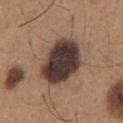notes=no biopsy performed (imaged during a skin exam); body site=the chest; TBP lesion metrics=a border-irregularity rating of about 1.5/10, a color-variation rating of about 6/10, and radial color variation of about 1.5; subject=male, about 65 years old; illumination=white-light; acquisition=~15 mm tile from a whole-body skin photo.